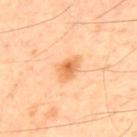| field | value |
|---|---|
| follow-up | catalogued during a skin exam; not biopsied |
| imaging modality | ~15 mm tile from a whole-body skin photo |
| location | the upper back |
| diameter | about 3.5 mm |
| lighting | cross-polarized |
| automated lesion analysis | a footprint of about 6 mm², an eccentricity of roughly 0.75, and a symmetry-axis asymmetry near 0.3; a lesion color around L≈68 a*≈25 b*≈44 in CIELAB, roughly 11 lightness units darker than nearby skin, and a normalized border contrast of about 7.5; a border-irregularity index near 3/10, a color-variation rating of about 4.5/10, and a peripheral color-asymmetry measure near 1; a nevus-likeness score of about 90/100 and lesion-presence confidence of about 100/100 |
| patient | male, approximately 60 years of age |